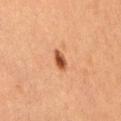Impression:
Part of a total-body skin-imaging series; this lesion was reviewed on a skin check and was not flagged for biopsy.
Acquisition and patient details:
A 15 mm close-up tile from a total-body photography series done for melanoma screening. A female subject aged 38–42. Measured at roughly 2.5 mm in maximum diameter. The lesion is on the right thigh. An algorithmic analysis of the crop reported a lesion area of about 3.5 mm², an eccentricity of roughly 0.75, and two-axis asymmetry of about 0.2. The analysis additionally found a nevus-likeness score of about 100/100 and lesion-presence confidence of about 100/100.